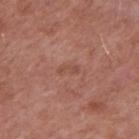workup=catalogued during a skin exam; not biopsied
image source=total-body-photography crop, ~15 mm field of view
subject=male, in their mid-50s
anatomic site=the right upper arm